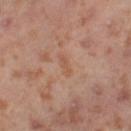Acquisition and patient details:
The lesion is on the left thigh. A female patient about 55 years old. A lesion tile, about 15 mm wide, cut from a 3D total-body photograph.